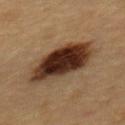This lesion was catalogued during total-body skin photography and was not selected for biopsy.
Captured under cross-polarized illumination.
On the mid back.
The lesion's longest dimension is about 7 mm.
A 15 mm close-up extracted from a 3D total-body photography capture.
The lesion-visualizer software estimated an outline eccentricity of about 0.75 (0 = round, 1 = elongated) and a symmetry-axis asymmetry near 0.15. It also reported a mean CIELAB color near L≈27 a*≈17 b*≈24 and a normalized border contrast of about 16.5. It also reported a classifier nevus-likeness of about 100/100 and lesion-presence confidence of about 100/100.
A female subject aged 58 to 62.